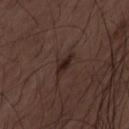<lesion>
<image>
  <source>total-body photography crop</source>
  <field_of_view_mm>15</field_of_view_mm>
</image>
<lesion_size>
  <long_diameter_mm_approx>3.0</long_diameter_mm_approx>
</lesion_size>
<lighting>white-light</lighting>
<site>leg</site>
<patient>
  <sex>male</sex>
  <age_approx>50</age_approx>
</patient>
</lesion>